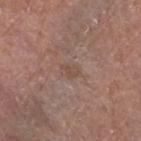Image and clinical context:
The lesion is located on the right lower leg. A lesion tile, about 15 mm wide, cut from a 3D total-body photograph. The subject is a female approximately 80 years of age.